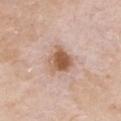Findings:
• follow-up · no biopsy performed (imaged during a skin exam)
• tile lighting · white-light
• automated metrics · a border-irregularity index near 3/10, internal color variation of about 5.5 on a 0–10 scale, and radial color variation of about 1.5
• body site · the chest
• patient · male, in their 70s
• image source · ~15 mm crop, total-body skin-cancer survey